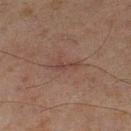Q: Is there a histopathology result?
A: no biopsy performed (imaged during a skin exam)
Q: Lesion size?
A: ≈3 mm
Q: What kind of image is this?
A: 15 mm crop, total-body photography
Q: Where on the body is the lesion?
A: the left lower leg
Q: What are the patient's age and sex?
A: male, roughly 45 years of age
Q: What lighting was used for the tile?
A: cross-polarized illumination